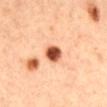A 15 mm close-up extracted from a 3D total-body photography capture. Captured under cross-polarized illumination. The lesion is on the mid back. The total-body-photography lesion software estimated an average lesion color of about L≈55 a*≈29 b*≈37 (CIELAB) and a normalized border contrast of about 13.5. It also reported a border-irregularity rating of about 1.5/10, a within-lesion color-variation index near 5/10, and radial color variation of about 1.5. The subject is a female in their mid- to late 40s. Measured at roughly 2.5 mm in maximum diameter.